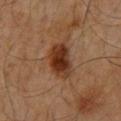Notes:
* follow-up: imaged on a skin check; not biopsied
* site: the chest
* lighting: cross-polarized
* diameter: ≈4.5 mm
* subject: male, roughly 60 years of age
* image source: 15 mm crop, total-body photography